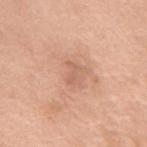• follow-up · total-body-photography surveillance lesion; no biopsy
• patient · female, aged approximately 75
• imaging modality · ~15 mm tile from a whole-body skin photo
• lesion size · about 2.5 mm
• tile lighting · white-light
• site · the mid back